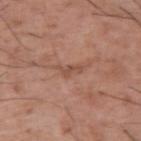The lesion was tiled from a total-body skin photograph and was not biopsied. A lesion tile, about 15 mm wide, cut from a 3D total-body photograph. On the right upper arm. A male subject, aged 53–57. The lesion's longest dimension is about 3 mm. Captured under white-light illumination.